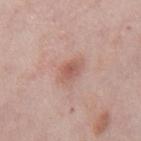Assessment: Imaged during a routine full-body skin examination; the lesion was not biopsied and no histopathology is available. Context: Located on the mid back. A region of skin cropped from a whole-body photographic capture, roughly 15 mm wide. The lesion's longest dimension is about 2.5 mm. A male subject, aged approximately 75. An algorithmic analysis of the crop reported a lesion area of about 4 mm², an outline eccentricity of about 0.65 (0 = round, 1 = elongated), and a symmetry-axis asymmetry near 0.2. The software also gave a nevus-likeness score of about 55/100 and lesion-presence confidence of about 100/100.